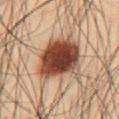Assessment:
No biopsy was performed on this lesion — it was imaged during a full skin examination and was not determined to be concerning.
Context:
Imaged with cross-polarized lighting. The recorded lesion diameter is about 6 mm. An algorithmic analysis of the crop reported an area of roughly 24 mm², a shape eccentricity near 0.6, and a shape-asymmetry score of about 0.25 (0 = symmetric). A male patient, aged approximately 55. From the abdomen. Cropped from a total-body skin-imaging series; the visible field is about 15 mm.